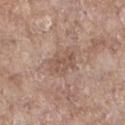Impression: Recorded during total-body skin imaging; not selected for excision or biopsy.